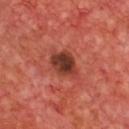No biopsy was performed on this lesion — it was imaged during a full skin examination and was not determined to be concerning. The recorded lesion diameter is about 4.5 mm. A 15 mm crop from a total-body photograph taken for skin-cancer surveillance. On the chest. A male patient, in their mid-60s. Imaged with cross-polarized lighting.